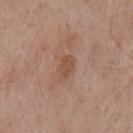Notes:
– biopsy status: no biopsy performed (imaged during a skin exam)
– location: the mid back
– image source: total-body-photography crop, ~15 mm field of view
– tile lighting: white-light
– patient: male, aged 53–57